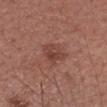Part of a total-body skin-imaging series; this lesion was reviewed on a skin check and was not flagged for biopsy.
On the right forearm.
Automated image analysis of the tile measured a lesion area of about 5 mm², an eccentricity of roughly 0.7, and a shape-asymmetry score of about 0.25 (0 = symmetric). It also reported an average lesion color of about L≈42 a*≈24 b*≈25 (CIELAB), about 8 CIELAB-L* units darker than the surrounding skin, and a normalized border contrast of about 6.5. It also reported border irregularity of about 2.5 on a 0–10 scale, a color-variation rating of about 3.5/10, and radial color variation of about 1.
A female patient, aged 53 to 57.
This is a white-light tile.
A region of skin cropped from a whole-body photographic capture, roughly 15 mm wide.
The recorded lesion diameter is about 3 mm.